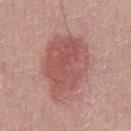The lesion was photographed on a routine skin check and not biopsied; there is no pathology result.